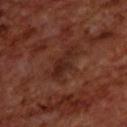follow-up: catalogued during a skin exam; not biopsied
patient: male, in their 70s
site: the upper back
tile lighting: cross-polarized
automated lesion analysis: an average lesion color of about L≈24 a*≈21 b*≈24 (CIELAB), a lesion–skin lightness drop of about 6, and a normalized lesion–skin contrast near 7
image: 15 mm crop, total-body photography
diameter: ~4.5 mm (longest diameter)